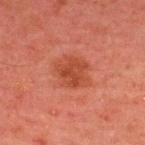Recorded during total-body skin imaging; not selected for excision or biopsy.
Captured under cross-polarized illumination.
Cropped from a total-body skin-imaging series; the visible field is about 15 mm.
The lesion is located on the upper back.
A male subject, aged around 45.